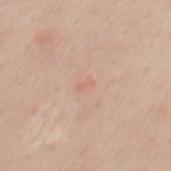Findings:
• workup · imaged on a skin check; not biopsied
• body site · the mid back
• image source · 15 mm crop, total-body photography
• patient · male, aged approximately 35